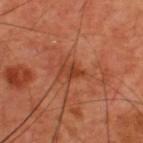Case summary:
– workup: no biopsy performed (imaged during a skin exam)
– image: 15 mm crop, total-body photography
– illumination: cross-polarized illumination
– TBP lesion metrics: an area of roughly 4 mm², a shape eccentricity near 0.8, and two-axis asymmetry of about 0.3; lesion-presence confidence of about 100/100
– site: the upper back
– patient: male, aged 58 to 62
– lesion size: ≈3 mm